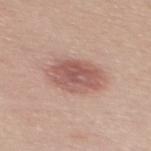Captured during whole-body skin photography for melanoma surveillance; the lesion was not biopsied. The subject is a female roughly 40 years of age. A close-up tile cropped from a whole-body skin photograph, about 15 mm across. From the mid back.